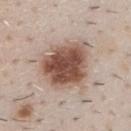Case summary:
• follow-up — total-body-photography surveillance lesion; no biopsy
• imaging modality — total-body-photography crop, ~15 mm field of view
• body site — the chest
• subject — male, aged approximately 50
• image-analysis metrics — a border-irregularity index near 2.5/10 and a color-variation rating of about 6/10; a lesion-detection confidence of about 100/100
• diameter — ~6 mm (longest diameter)
• tile lighting — white-light illumination